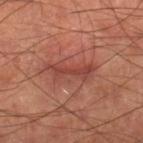Part of a total-body skin-imaging series; this lesion was reviewed on a skin check and was not flagged for biopsy.